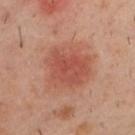  image:
    source: total-body photography crop
    field_of_view_mm: 15
  site: upper back
  lesion_size:
    long_diameter_mm_approx: 6.0
  automated_metrics:
    area_mm2_approx: 26.0
    eccentricity: 0.4
    nevus_likeness_0_100: 80
    lesion_detection_confidence_0_100: 100
  lighting: cross-polarized
  patient:
    sex: male
    age_approx: 40